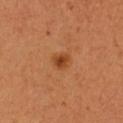<case>
  <biopsy_status>not biopsied; imaged during a skin examination</biopsy_status>
  <lesion_size>
    <long_diameter_mm_approx>2.5</long_diameter_mm_approx>
  </lesion_size>
  <lighting>cross-polarized</lighting>
  <patient>
    <sex>female</sex>
    <age_approx>40</age_approx>
  </patient>
  <image>
    <source>total-body photography crop</source>
    <field_of_view_mm>15</field_of_view_mm>
  </image>
  <automated_metrics>
    <area_mm2_approx>3.5</area_mm2_approx>
    <cielab_L>43</cielab_L>
    <cielab_a>27</cielab_a>
    <cielab_b>40</cielab_b>
    <border_irregularity_0_10>2.5</border_irregularity_0_10>
    <peripheral_color_asymmetry>0.5</peripheral_color_asymmetry>
  </automated_metrics>
  <site>chest</site>
</case>